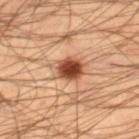Cropped from a total-body skin-imaging series; the visible field is about 15 mm. A male subject approximately 45 years of age. On the right lower leg. Automated tile analysis of the lesion measured a lesion color around L≈49 a*≈25 b*≈34 in CIELAB, a lesion–skin lightness drop of about 17, and a lesion-to-skin contrast of about 11.5 (normalized; higher = more distinct). It also reported border irregularity of about 2.5 on a 0–10 scale, a within-lesion color-variation index near 6.5/10, and peripheral color asymmetry of about 1.5. It also reported an automated nevus-likeness rating near 100 out of 100 and a lesion-detection confidence of about 100/100.